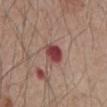biopsy status = total-body-photography surveillance lesion; no biopsy
image = ~15 mm crop, total-body skin-cancer survey
patient = male, aged around 80
anatomic site = the front of the torso